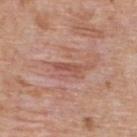subject — female, in their 40s | diameter — about 3.5 mm | anatomic site — the upper back | image — ~15 mm crop, total-body skin-cancer survey | lighting — white-light | image-analysis metrics — a lesion area of about 3.5 mm², an eccentricity of roughly 0.9, and two-axis asymmetry of about 0.45; a mean CIELAB color near L≈53 a*≈25 b*≈28 and a normalized border contrast of about 6; an automated nevus-likeness rating near 0 out of 100 and a detector confidence of about 80 out of 100 that the crop contains a lesion.